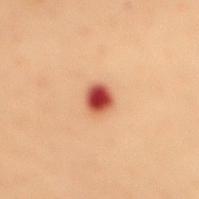Q: Was this lesion biopsied?
A: imaged on a skin check; not biopsied
Q: What kind of image is this?
A: 15 mm crop, total-body photography
Q: How was the tile lit?
A: cross-polarized illumination
Q: What is the anatomic site?
A: the mid back
Q: What are the patient's age and sex?
A: female, about 50 years old
Q: How large is the lesion?
A: ~2.5 mm (longest diameter)